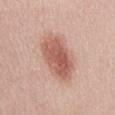  lesion_size:
    long_diameter_mm_approx: 7.0
  site: abdomen
  image:
    source: total-body photography crop
    field_of_view_mm: 15
  automated_metrics:
    area_mm2_approx: 19.0
    eccentricity: 0.85
    nevus_likeness_0_100: 100
    lesion_detection_confidence_0_100: 100
  patient:
    sex: female
    age_approx: 40